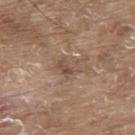Impression: The lesion was tiled from a total-body skin photograph and was not biopsied. Background: On the back. A close-up tile cropped from a whole-body skin photograph, about 15 mm across. A male patient, aged around 80.